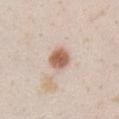follow-up: total-body-photography surveillance lesion; no biopsy | subject: female, aged around 25 | lesion size: about 2.5 mm | location: the chest | tile lighting: white-light | automated lesion analysis: an area of roughly 6 mm², an eccentricity of roughly 0.35, and a symmetry-axis asymmetry near 0.15; a mean CIELAB color near L≈61 a*≈20 b*≈31 and a normalized border contrast of about 10.5; a border-irregularity index near 1.5/10, internal color variation of about 3 on a 0–10 scale, and a peripheral color-asymmetry measure near 1 | acquisition: 15 mm crop, total-body photography.